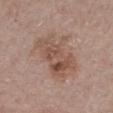The patient is a male aged 83 or older.
The lesion's longest dimension is about 6.5 mm.
The lesion is on the chest.
A 15 mm close-up extracted from a 3D total-body photography capture.